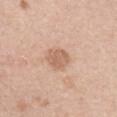The lesion was tiled from a total-body skin photograph and was not biopsied. A male patient roughly 45 years of age. A 15 mm crop from a total-body photograph taken for skin-cancer surveillance. On the right upper arm. The recorded lesion diameter is about 3 mm. The tile uses white-light illumination.